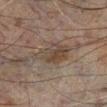Q: Was a biopsy performed?
A: catalogued during a skin exam; not biopsied
Q: Where on the body is the lesion?
A: the left lower leg
Q: What did automated image analysis measure?
A: a lesion area of about 12 mm² and a symmetry-axis asymmetry near 0.3; a lesion color around L≈32 a*≈10 b*≈19 in CIELAB and a lesion-to-skin contrast of about 6 (normalized; higher = more distinct); a peripheral color-asymmetry measure near 1.5
Q: How was this image acquired?
A: ~15 mm tile from a whole-body skin photo
Q: Who is the patient?
A: male, approximately 60 years of age